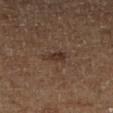Findings:
– workup — imaged on a skin check; not biopsied
– acquisition — total-body-photography crop, ~15 mm field of view
– automated metrics — a footprint of about 3.5 mm² and an eccentricity of roughly 0.85; an average lesion color of about L≈32 a*≈16 b*≈23 (CIELAB), roughly 8 lightness units darker than nearby skin, and a normalized border contrast of about 7.5; a border-irregularity index near 3.5/10 and internal color variation of about 2 on a 0–10 scale; a detector confidence of about 100 out of 100 that the crop contains a lesion
– tile lighting — cross-polarized illumination
– anatomic site — the right lower leg
– subject — male, aged approximately 65
– lesion diameter — ≈3 mm